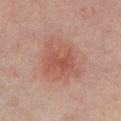No biopsy was performed on this lesion — it was imaged during a full skin examination and was not determined to be concerning.
The tile uses cross-polarized illumination.
An algorithmic analysis of the crop reported a lesion color around L≈43 a*≈20 b*≈23 in CIELAB, a lesion–skin lightness drop of about 6, and a normalized border contrast of about 5.5. The software also gave a border-irregularity index near 2.5/10 and internal color variation of about 3.5 on a 0–10 scale. The software also gave an automated nevus-likeness rating near 40 out of 100 and lesion-presence confidence of about 100/100.
A male patient aged 28–32.
This image is a 15 mm lesion crop taken from a total-body photograph.
From the chest.